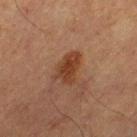• site: the right thigh
• image-analysis metrics: a lesion–skin lightness drop of about 8; a nevus-likeness score of about 90/100 and a detector confidence of about 100 out of 100 that the crop contains a lesion
• lesion diameter: ~4 mm (longest diameter)
• subject: male, approximately 65 years of age
• imaging modality: total-body-photography crop, ~15 mm field of view